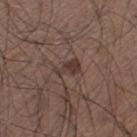Clinical impression: The lesion was photographed on a routine skin check and not biopsied; there is no pathology result. Clinical summary: Cropped from a total-body skin-imaging series; the visible field is about 15 mm. On the left thigh. Measured at roughly 3 mm in maximum diameter. The subject is a male in their mid-50s. This is a white-light tile.